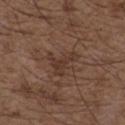No biopsy was performed on this lesion — it was imaged during a full skin examination and was not determined to be concerning.
Approximately 4.5 mm at its widest.
A roughly 15 mm field-of-view crop from a total-body skin photograph.
The patient is a male in their 50s.
Located on the chest.